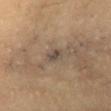This lesion was catalogued during total-body skin photography and was not selected for biopsy.
This image is a 15 mm lesion crop taken from a total-body photograph.
The recorded lesion diameter is about 3 mm.
Imaged with cross-polarized lighting.
The lesion is on the left upper arm.
A female subject, aged around 35.